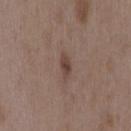The lesion was photographed on a routine skin check and not biopsied; there is no pathology result.
A 15 mm crop from a total-body photograph taken for skin-cancer surveillance.
The tile uses white-light illumination.
The lesion is located on the mid back.
Longest diameter approximately 2.5 mm.
A female subject in their mid-30s.
Automated tile analysis of the lesion measured an eccentricity of roughly 0.85 and two-axis asymmetry of about 0.3. The analysis additionally found a lesion color around L≈42 a*≈16 b*≈22 in CIELAB, a lesion–skin lightness drop of about 9, and a normalized lesion–skin contrast near 7.5. The software also gave a border-irregularity index near 2.5/10, internal color variation of about 2.5 on a 0–10 scale, and peripheral color asymmetry of about 1. It also reported an automated nevus-likeness rating near 35 out of 100 and a detector confidence of about 100 out of 100 that the crop contains a lesion.